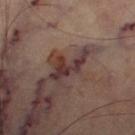No biopsy was performed on this lesion — it was imaged during a full skin examination and was not determined to be concerning. This image is a 15 mm lesion crop taken from a total-body photograph. The lesion is on the right thigh.